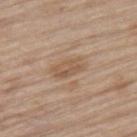workup: catalogued during a skin exam; not biopsied
TBP lesion metrics: a footprint of about 5.5 mm², an outline eccentricity of about 0.85 (0 = round, 1 = elongated), and a symmetry-axis asymmetry near 0.25
tile lighting: white-light
subject: male, aged approximately 70
anatomic site: the right thigh
lesion size: ~3.5 mm (longest diameter)
image: 15 mm crop, total-body photography